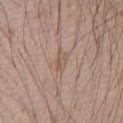The lesion was tiled from a total-body skin photograph and was not biopsied.
A male patient, aged 63–67.
About 2.5 mm across.
Imaged with white-light lighting.
Automated image analysis of the tile measured a lesion–skin lightness drop of about 6. And it measured lesion-presence confidence of about 100/100.
A roughly 15 mm field-of-view crop from a total-body skin photograph.
The lesion is located on the chest.